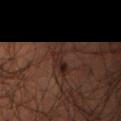Clinical impression: Part of a total-body skin-imaging series; this lesion was reviewed on a skin check and was not flagged for biopsy. Background: Imaged with cross-polarized lighting. A roughly 15 mm field-of-view crop from a total-body skin photograph. A male subject approximately 50 years of age. Located on the right thigh.